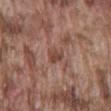Assessment:
No biopsy was performed on this lesion — it was imaged during a full skin examination and was not determined to be concerning.
Clinical summary:
On the lower back. A male subject aged 73–77. Cropped from a total-body skin-imaging series; the visible field is about 15 mm.